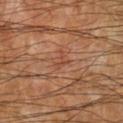A 15 mm crop from a total-body photograph taken for skin-cancer surveillance.
Captured under cross-polarized illumination.
The lesion is located on the left lower leg.
The lesion-visualizer software estimated a footprint of about 2.5 mm², a shape eccentricity near 0.8, and a shape-asymmetry score of about 0.7 (0 = symmetric). The analysis additionally found an automated nevus-likeness rating near 0 out of 100 and a detector confidence of about 95 out of 100 that the crop contains a lesion.
The subject is a male aged around 60.